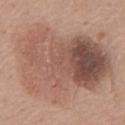Findings:
- workup · imaged on a skin check; not biopsied
- tile lighting · white-light
- patient · female, in their 60s
- automated lesion analysis · a lesion area of about 75 mm², an outline eccentricity of about 0.7 (0 = round, 1 = elongated), and two-axis asymmetry of about 0.3; a lesion color around L≈54 a*≈19 b*≈26 in CIELAB, roughly 11 lightness units darker than nearby skin, and a normalized lesion–skin contrast near 7.5; border irregularity of about 4.5 on a 0–10 scale, internal color variation of about 9.5 on a 0–10 scale, and peripheral color asymmetry of about 4; a lesion-detection confidence of about 100/100
- anatomic site · the chest
- acquisition · total-body-photography crop, ~15 mm field of view